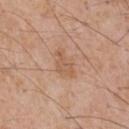  biopsy_status: not biopsied; imaged during a skin examination
  lighting: white-light
  patient:
    sex: male
    age_approx: 60
  image:
    source: total-body photography crop
    field_of_view_mm: 15
  site: front of the torso
  lesion_size:
    long_diameter_mm_approx: 3.5